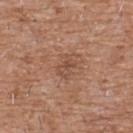Captured during whole-body skin photography for melanoma surveillance; the lesion was not biopsied.
Located on the chest.
The tile uses white-light illumination.
A male patient approximately 60 years of age.
The recorded lesion diameter is about 3 mm.
A 15 mm close-up tile from a total-body photography series done for melanoma screening.